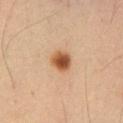Findings:
• follow-up: imaged on a skin check; not biopsied
• anatomic site: the abdomen
• image-analysis metrics: a footprint of about 6 mm² and an eccentricity of roughly 0.35; an average lesion color of about L≈52 a*≈22 b*≈36 (CIELAB), a lesion–skin lightness drop of about 15, and a lesion-to-skin contrast of about 10.5 (normalized; higher = more distinct); a border-irregularity index near 2/10 and a within-lesion color-variation index near 5/10; a lesion-detection confidence of about 100/100
• lighting: cross-polarized illumination
• patient: male, about 55 years old
• image: ~15 mm crop, total-body skin-cancer survey